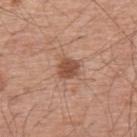Captured during whole-body skin photography for melanoma surveillance; the lesion was not biopsied. A close-up tile cropped from a whole-body skin photograph, about 15 mm across. Longest diameter approximately 2.5 mm. On the upper back. A male subject, aged 53 to 57.